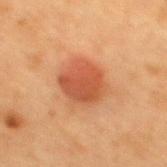Assessment: Imaged during a routine full-body skin examination; the lesion was not biopsied and no histopathology is available. Image and clinical context: A female patient aged approximately 40. About 4 mm across. Imaged with cross-polarized lighting. An algorithmic analysis of the crop reported a lesion area of about 14 mm², an outline eccentricity of about 0.15 (0 = round, 1 = elongated), and a shape-asymmetry score of about 0.15 (0 = symmetric). It also reported an average lesion color of about L≈47 a*≈27 b*≈34 (CIELAB) and a normalized lesion–skin contrast near 7.5. The analysis additionally found a classifier nevus-likeness of about 100/100 and a lesion-detection confidence of about 100/100. Located on the mid back. A 15 mm close-up extracted from a 3D total-body photography capture.